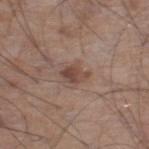No biopsy was performed on this lesion — it was imaged during a full skin examination and was not determined to be concerning. Captured under white-light illumination. On the left lower leg. The subject is a male aged approximately 70. This image is a 15 mm lesion crop taken from a total-body photograph. The recorded lesion diameter is about 2.5 mm. Automated image analysis of the tile measured an area of roughly 5 mm², an outline eccentricity of about 0.55 (0 = round, 1 = elongated), and two-axis asymmetry of about 0.3. And it measured a lesion color around L≈46 a*≈18 b*≈24 in CIELAB, a lesion–skin lightness drop of about 9, and a normalized border contrast of about 7.5. The software also gave a nevus-likeness score of about 35/100 and lesion-presence confidence of about 100/100.